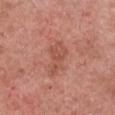workup = no biopsy performed (imaged during a skin exam) | anatomic site = the chest | subject = female, aged 58–62 | lighting = white-light illumination | acquisition = total-body-photography crop, ~15 mm field of view.